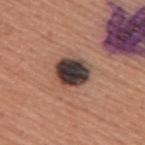Assessment:
Imaged during a routine full-body skin examination; the lesion was not biopsied and no histopathology is available.